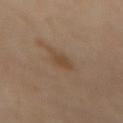<case>
  <biopsy_status>not biopsied; imaged during a skin examination</biopsy_status>
  <patient>
    <sex>female</sex>
    <age_approx>55</age_approx>
  </patient>
  <lighting>cross-polarized</lighting>
  <lesion_size>
    <long_diameter_mm_approx>3.0</long_diameter_mm_approx>
  </lesion_size>
  <image>
    <source>total-body photography crop</source>
    <field_of_view_mm>15</field_of_view_mm>
  </image>
  <site>abdomen</site>
</case>